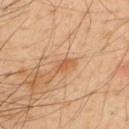Clinical summary: The lesion is on the upper back. A 15 mm crop from a total-body photograph taken for skin-cancer surveillance. The subject is a male aged 48–52.